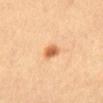The lesion was photographed on a routine skin check and not biopsied; there is no pathology result. A 15 mm close-up extracted from a 3D total-body photography capture. Measured at roughly 2.5 mm in maximum diameter. A male patient aged approximately 20. The lesion is on the abdomen.